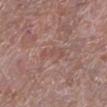Context:
Cropped from a total-body skin-imaging series; the visible field is about 15 mm. The subject is a male in their mid-60s. The lesion is located on the left lower leg. Longest diameter approximately 3.5 mm. Captured under white-light illumination.